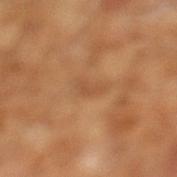notes = no biopsy performed (imaged during a skin exam)
image = 15 mm crop, total-body photography
size = ~3 mm (longest diameter)
illumination = cross-polarized illumination
subject = male, about 65 years old